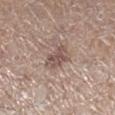biopsy status = total-body-photography surveillance lesion; no biopsy | tile lighting = white-light | acquisition = total-body-photography crop, ~15 mm field of view | subject = male, aged 63–67 | lesion size = ≈3.5 mm | anatomic site = the right lower leg.